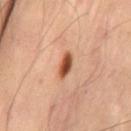biopsy status — imaged on a skin check; not biopsied | imaging modality — 15 mm crop, total-body photography | anatomic site — the lower back | subject — male, aged 53 to 57.